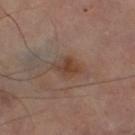Impression:
This lesion was catalogued during total-body skin photography and was not selected for biopsy.
Image and clinical context:
From the leg. This is a cross-polarized tile. A lesion tile, about 15 mm wide, cut from a 3D total-body photograph. Measured at roughly 3 mm in maximum diameter.